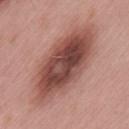Captured during whole-body skin photography for melanoma surveillance; the lesion was not biopsied.
Captured under white-light illumination.
A close-up tile cropped from a whole-body skin photograph, about 15 mm across.
The lesion is located on the lower back.
Automated tile analysis of the lesion measured a lesion area of about 41 mm² and two-axis asymmetry of about 0.1.
A male patient, roughly 55 years of age.
Longest diameter approximately 11 mm.